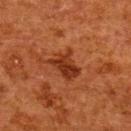biopsy status = imaged on a skin check; not biopsied | image = 15 mm crop, total-body photography | body site = the upper back | diameter = ≈4 mm | subject = female, aged approximately 50 | lighting = cross-polarized.